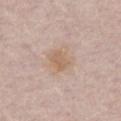Assessment: Imaged during a routine full-body skin examination; the lesion was not biopsied and no histopathology is available. Background: Imaged with white-light lighting. The subject is a male aged 63 to 67. The lesion is located on the front of the torso. A 15 mm close-up extracted from a 3D total-body photography capture. Automated image analysis of the tile measured a footprint of about 8 mm², an outline eccentricity of about 0.55 (0 = round, 1 = elongated), and a symmetry-axis asymmetry near 0.2.